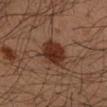Notes:
• biopsy status: imaged on a skin check; not biopsied
• location: the arm
• image: ~15 mm crop, total-body skin-cancer survey
• patient: male, roughly 35 years of age